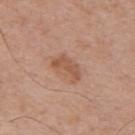Q: Is there a histopathology result?
A: total-body-photography surveillance lesion; no biopsy
Q: Illumination type?
A: white-light illumination
Q: What is the imaging modality?
A: total-body-photography crop, ~15 mm field of view
Q: Lesion location?
A: the upper back
Q: What are the patient's age and sex?
A: male, about 55 years old
Q: How large is the lesion?
A: about 4 mm